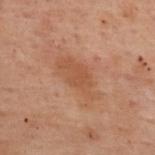Imaged during a routine full-body skin examination; the lesion was not biopsied and no histopathology is available. About 5.5 mm across. Captured under cross-polarized illumination. An algorithmic analysis of the crop reported internal color variation of about 1.5 on a 0–10 scale and a peripheral color-asymmetry measure near 0.5. A roughly 15 mm field-of-view crop from a total-body skin photograph. Located on the upper back. A female patient, in their mid- to late 50s.